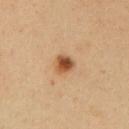{
  "biopsy_status": "not biopsied; imaged during a skin examination",
  "image": {
    "source": "total-body photography crop",
    "field_of_view_mm": 15
  },
  "lesion_size": {
    "long_diameter_mm_approx": 2.5
  },
  "patient": {
    "sex": "male",
    "age_approx": 50
  },
  "site": "abdomen",
  "lighting": "cross-polarized"
}